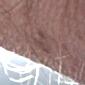Q: Is there a histopathology result?
A: catalogued during a skin exam; not biopsied
Q: What is the lesion's diameter?
A: ~4 mm (longest diameter)
Q: Where on the body is the lesion?
A: the right forearm
Q: What kind of image is this?
A: ~15 mm tile from a whole-body skin photo
Q: Who is the patient?
A: male, about 60 years old
Q: How was the tile lit?
A: white-light